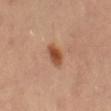Recorded during total-body skin imaging; not selected for excision or biopsy. Cropped from a total-body skin-imaging series; the visible field is about 15 mm. The recorded lesion diameter is about 3.5 mm. A female subject roughly 35 years of age. This is a cross-polarized tile. On the lower back.